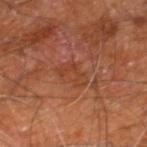Part of a total-body skin-imaging series; this lesion was reviewed on a skin check and was not flagged for biopsy. Automated image analysis of the tile measured a lesion color around L≈40 a*≈25 b*≈33 in CIELAB, roughly 5 lightness units darker than nearby skin, and a normalized lesion–skin contrast near 5. The recorded lesion diameter is about 3.5 mm. This is a cross-polarized tile. A lesion tile, about 15 mm wide, cut from a 3D total-body photograph. A male subject, aged around 60. On the right leg.